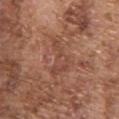Imaged during a routine full-body skin examination; the lesion was not biopsied and no histopathology is available.
The total-body-photography lesion software estimated a footprint of about 7 mm² and an outline eccentricity of about 0.85 (0 = round, 1 = elongated). It also reported a color-variation rating of about 1/10 and a peripheral color-asymmetry measure near 0.5. And it measured a nevus-likeness score of about 0/100 and a lesion-detection confidence of about 55/100.
A male patient, approximately 75 years of age.
From the upper back.
Approximately 4.5 mm at its widest.
The tile uses white-light illumination.
A close-up tile cropped from a whole-body skin photograph, about 15 mm across.